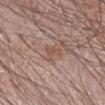Case summary:
– notes — catalogued during a skin exam; not biopsied
– subject — male, in their mid- to late 60s
– tile lighting — white-light illumination
– acquisition — 15 mm crop, total-body photography
– location — the right forearm